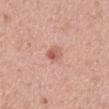The tile uses white-light illumination. The lesion is on the mid back. A region of skin cropped from a whole-body photographic capture, roughly 15 mm wide. Longest diameter approximately 2.5 mm. The patient is a male aged 38 to 42. The lesion-visualizer software estimated an area of roughly 3.5 mm² and a shape-asymmetry score of about 0.2 (0 = symmetric). The software also gave a lesion color around L≈59 a*≈27 b*≈28 in CIELAB, about 10 CIELAB-L* units darker than the surrounding skin, and a lesion-to-skin contrast of about 6.5 (normalized; higher = more distinct). The analysis additionally found an automated nevus-likeness rating near 40 out of 100 and a detector confidence of about 100 out of 100 that the crop contains a lesion.